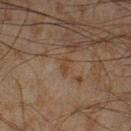follow-up = catalogued during a skin exam; not biopsied | tile lighting = cross-polarized illumination | subject = male, aged 43 to 47 | anatomic site = the right lower leg | TBP lesion metrics = a border-irregularity rating of about 3.5/10 and internal color variation of about 0 on a 0–10 scale; a classifier nevus-likeness of about 0/100 and a detector confidence of about 70 out of 100 that the crop contains a lesion | lesion diameter = ~2.5 mm (longest diameter) | imaging modality = ~15 mm tile from a whole-body skin photo.